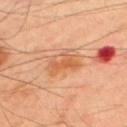Assessment:
This lesion was catalogued during total-body skin photography and was not selected for biopsy.